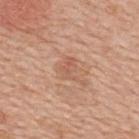Captured during whole-body skin photography for melanoma surveillance; the lesion was not biopsied.
The lesion-visualizer software estimated an area of roughly 4 mm² and an outline eccentricity of about 0.7 (0 = round, 1 = elongated). The software also gave a border-irregularity index near 5/10, a within-lesion color-variation index near 1.5/10, and a peripheral color-asymmetry measure near 0.5.
Approximately 2.5 mm at its widest.
On the upper back.
This image is a 15 mm lesion crop taken from a total-body photograph.
A female subject about 50 years old.
The tile uses white-light illumination.